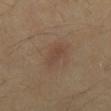notes: no biopsy performed (imaged during a skin exam)
imaging modality: total-body-photography crop, ~15 mm field of view
body site: the back
patient: male, aged 38–42